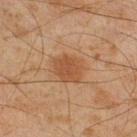Clinical summary: A male subject in their mid- to late 40s. Longest diameter approximately 3.5 mm. Imaged with cross-polarized lighting. A 15 mm crop from a total-body photograph taken for skin-cancer surveillance. Located on the right lower leg.